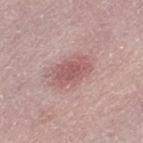Q: Was this lesion biopsied?
A: no biopsy performed (imaged during a skin exam)
Q: Who is the patient?
A: female, in their mid- to late 60s
Q: Where on the body is the lesion?
A: the leg
Q: What kind of image is this?
A: ~15 mm crop, total-body skin-cancer survey